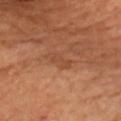Captured during whole-body skin photography for melanoma surveillance; the lesion was not biopsied. A 15 mm close-up tile from a total-body photography series done for melanoma screening. The tile uses cross-polarized illumination. A male subject aged 68 to 72. On the front of the torso. The lesion's longest dimension is about 3 mm.